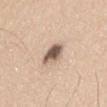No biopsy was performed on this lesion — it was imaged during a full skin examination and was not determined to be concerning. The recorded lesion diameter is about 3 mm. A region of skin cropped from a whole-body photographic capture, roughly 15 mm wide. A male subject aged 38 to 42. From the lower back. The total-body-photography lesion software estimated a classifier nevus-likeness of about 60/100 and a detector confidence of about 100 out of 100 that the crop contains a lesion.